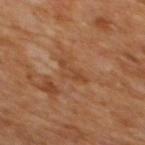  biopsy_status: not biopsied; imaged during a skin examination
  site: mid back
  lesion_size:
    long_diameter_mm_approx: 3.5
  patient:
    sex: male
    age_approx: 65
  image:
    source: total-body photography crop
    field_of_view_mm: 15
  lighting: cross-polarized